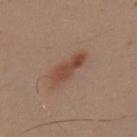{"site": "upper back", "lesion_size": {"long_diameter_mm_approx": 5.0}, "patient": {"sex": "male", "age_approx": 30}, "image": {"source": "total-body photography crop", "field_of_view_mm": 15}}